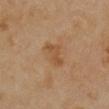The lesion was tiled from a total-body skin photograph and was not biopsied.
A female subject aged 33–37.
The lesion is located on the front of the torso.
Cropped from a whole-body photographic skin survey; the tile spans about 15 mm.
Automated tile analysis of the lesion measured a lesion area of about 5.5 mm², an eccentricity of roughly 0.85, and two-axis asymmetry of about 0.35. And it measured a color-variation rating of about 2/10 and radial color variation of about 0.5.